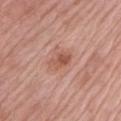Notes:
• imaging modality — 15 mm crop, total-body photography
• lesion size — ~3 mm (longest diameter)
• lighting — white-light illumination
• site — the left upper arm
• TBP lesion metrics — an average lesion color of about L≈55 a*≈24 b*≈30 (CIELAB), roughly 10 lightness units darker than nearby skin, and a normalized lesion–skin contrast near 7; a classifier nevus-likeness of about 40/100 and a lesion-detection confidence of about 100/100
• subject — male, aged around 70